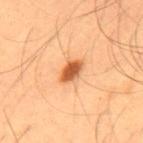– follow-up — catalogued during a skin exam; not biopsied